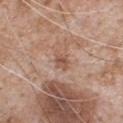workup = total-body-photography surveillance lesion; no biopsy | image source = 15 mm crop, total-body photography | patient = male, about 65 years old | lighting = white-light | anatomic site = the chest.